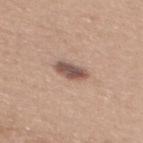follow-up: total-body-photography surveillance lesion; no biopsy
image source: total-body-photography crop, ~15 mm field of view
site: the mid back
TBP lesion metrics: an outline eccentricity of about 0.85 (0 = round, 1 = elongated) and two-axis asymmetry of about 0.2; an automated nevus-likeness rating near 70 out of 100 and a detector confidence of about 100 out of 100 that the crop contains a lesion
illumination: white-light
subject: female, in their mid- to late 30s